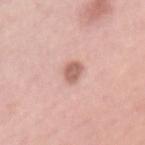{
  "biopsy_status": "not biopsied; imaged during a skin examination",
  "site": "mid back",
  "patient": {
    "sex": "female",
    "age_approx": 65
  },
  "lesion_size": {
    "long_diameter_mm_approx": 3.0
  },
  "automated_metrics": {
    "eccentricity": 0.85,
    "shape_asymmetry": 0.2,
    "cielab_L": 61,
    "cielab_a": 23,
    "cielab_b": 26
  },
  "image": {
    "source": "total-body photography crop",
    "field_of_view_mm": 15
  }
}